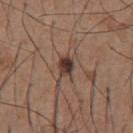Imaged during a routine full-body skin examination; the lesion was not biopsied and no histopathology is available.
This is a white-light tile.
The patient is a male about 55 years old.
The lesion's longest dimension is about 2.5 mm.
On the chest.
A 15 mm close-up tile from a total-body photography series done for melanoma screening.